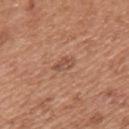Notes:
- subject · male, in their mid-60s
- location · the mid back
- automated metrics · a footprint of about 3.5 mm² and two-axis asymmetry of about 0.3; roughly 8 lightness units darker than nearby skin and a normalized lesion–skin contrast near 6; border irregularity of about 2.5 on a 0–10 scale, a color-variation rating of about 3.5/10, and radial color variation of about 1.5; a nevus-likeness score of about 15/100
- image · total-body-photography crop, ~15 mm field of view
- diameter · ≈2.5 mm
- tile lighting · white-light illumination